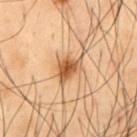The lesion was photographed on a routine skin check and not biopsied; there is no pathology result. A male subject, aged approximately 55. An algorithmic analysis of the crop reported a mean CIELAB color near L≈47 a*≈19 b*≈33, about 12 CIELAB-L* units darker than the surrounding skin, and a normalized lesion–skin contrast near 9. The software also gave a classifier nevus-likeness of about 95/100. A close-up tile cropped from a whole-body skin photograph, about 15 mm across. About 3.5 mm across. On the chest. Imaged with cross-polarized lighting.